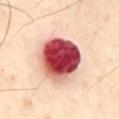| field | value |
|---|---|
| workup | catalogued during a skin exam; not biopsied |
| image | 15 mm crop, total-body photography |
| subject | male, in their mid-60s |
| illumination | cross-polarized illumination |
| TBP lesion metrics | a mean CIELAB color near L≈49 a*≈35 b*≈25, a lesion–skin lightness drop of about 28, and a normalized lesion–skin contrast near 17; a border-irregularity rating of about 1/10, a within-lesion color-variation index near 10/10, and radial color variation of about 4 |
| site | the chest |